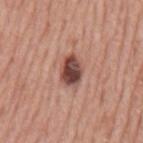Notes:
* follow-up · no biopsy performed (imaged during a skin exam)
* site · the mid back
* image · ~15 mm crop, total-body skin-cancer survey
* automated lesion analysis · an area of roughly 7.5 mm², an outline eccentricity of about 0.7 (0 = round, 1 = elongated), and a symmetry-axis asymmetry near 0.15; a border-irregularity rating of about 1.5/10, internal color variation of about 6.5 on a 0–10 scale, and a peripheral color-asymmetry measure near 2.5; a lesion-detection confidence of about 100/100
* subject · male, aged around 75